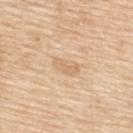Q: Is there a histopathology result?
A: catalogued during a skin exam; not biopsied
Q: What are the patient's age and sex?
A: female, about 55 years old
Q: What kind of image is this?
A: ~15 mm tile from a whole-body skin photo
Q: What is the anatomic site?
A: the upper back
Q: How was the tile lit?
A: white-light illumination